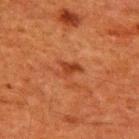This lesion was catalogued during total-body skin photography and was not selected for biopsy. A region of skin cropped from a whole-body photographic capture, roughly 15 mm wide. On the upper back. The patient is a male approximately 60 years of age. Longest diameter approximately 3 mm. Captured under cross-polarized illumination.